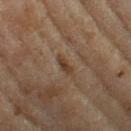Captured during whole-body skin photography for melanoma surveillance; the lesion was not biopsied.
A female subject aged 58–62.
The total-body-photography lesion software estimated a border-irregularity rating of about 3/10. It also reported a nevus-likeness score of about 5/100.
A 15 mm crop from a total-body photograph taken for skin-cancer surveillance.
The lesion is located on the left thigh.
Longest diameter approximately 3 mm.